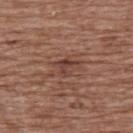| key | value |
|---|---|
| follow-up | catalogued during a skin exam; not biopsied |
| image | ~15 mm tile from a whole-body skin photo |
| patient | female, about 75 years old |
| image-analysis metrics | an area of roughly 4 mm², a shape eccentricity near 0.65, and a shape-asymmetry score of about 0.55 (0 = symmetric); a mean CIELAB color near L≈41 a*≈21 b*≈25, a lesion–skin lightness drop of about 8, and a lesion-to-skin contrast of about 7 (normalized; higher = more distinct); a within-lesion color-variation index near 4/10 and radial color variation of about 1.5; a classifier nevus-likeness of about 0/100 |
| anatomic site | the upper back |
| diameter | about 3 mm |
| illumination | white-light illumination |